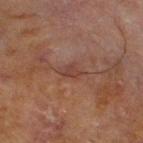notes: no biopsy performed (imaged during a skin exam) | location: the leg | image: 15 mm crop, total-body photography | diameter: ≈3 mm | lighting: cross-polarized | subject: male, aged 68–72.